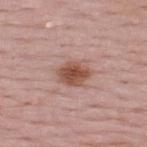Impression: This lesion was catalogued during total-body skin photography and was not selected for biopsy. Clinical summary: On the upper back. A close-up tile cropped from a whole-body skin photograph, about 15 mm across. The tile uses white-light illumination. The patient is a male approximately 50 years of age. Measured at roughly 4 mm in maximum diameter. Automated tile analysis of the lesion measured a mean CIELAB color near L≈52 a*≈22 b*≈27 and a lesion-to-skin contrast of about 9 (normalized; higher = more distinct). It also reported a border-irregularity rating of about 2/10 and a color-variation rating of about 4/10. It also reported a classifier nevus-likeness of about 95/100 and lesion-presence confidence of about 100/100.